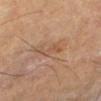Imaged during a routine full-body skin examination; the lesion was not biopsied and no histopathology is available.
The recorded lesion diameter is about 4 mm.
The lesion is on the left lower leg.
A male subject, in their 60s.
Imaged with cross-polarized lighting.
A 15 mm close-up extracted from a 3D total-body photography capture.